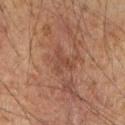Assessment:
This lesion was catalogued during total-body skin photography and was not selected for biopsy.
Context:
The recorded lesion diameter is about 3 mm. Captured under cross-polarized illumination. Automated tile analysis of the lesion measured a border-irregularity index near 8/10, a within-lesion color-variation index near 0/10, and peripheral color asymmetry of about 0. And it measured a classifier nevus-likeness of about 0/100 and lesion-presence confidence of about 100/100. A male subject about 50 years old. A region of skin cropped from a whole-body photographic capture, roughly 15 mm wide. The lesion is located on the right upper arm.